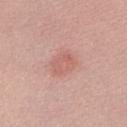Assessment: No biopsy was performed on this lesion — it was imaged during a full skin examination and was not determined to be concerning.